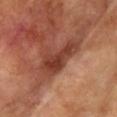biopsy_status: not biopsied; imaged during a skin examination
lighting: cross-polarized
site: right upper arm
image:
  source: total-body photography crop
  field_of_view_mm: 15
patient:
  sex: female
  age_approx: 75